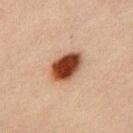{"biopsy_status": "not biopsied; imaged during a skin examination", "patient": {"sex": "male", "age_approx": 30}, "lesion_size": {"long_diameter_mm_approx": 4.0}, "image": {"source": "total-body photography crop", "field_of_view_mm": 15}, "site": "chest"}